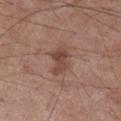The patient is a male aged around 55. A region of skin cropped from a whole-body photographic capture, roughly 15 mm wide. The lesion is on the right lower leg.